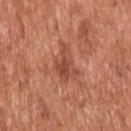The tile uses white-light illumination.
An algorithmic analysis of the crop reported an area of roughly 8.5 mm² and two-axis asymmetry of about 0.45. The software also gave roughly 9 lightness units darker than nearby skin and a normalized lesion–skin contrast near 6.5. And it measured a detector confidence of about 100 out of 100 that the crop contains a lesion.
Measured at roughly 4.5 mm in maximum diameter.
A male patient, aged 63 to 67.
Cropped from a whole-body photographic skin survey; the tile spans about 15 mm.
From the upper back.